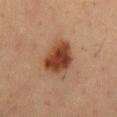Imaged during a routine full-body skin examination; the lesion was not biopsied and no histopathology is available. A 15 mm crop from a total-body photograph taken for skin-cancer surveillance. The tile uses cross-polarized illumination. Measured at roughly 4.5 mm in maximum diameter. A male patient aged around 65. On the abdomen.